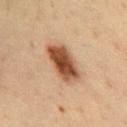Clinical impression: The lesion was photographed on a routine skin check and not biopsied; there is no pathology result.